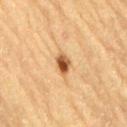Recorded during total-body skin imaging; not selected for excision or biopsy. A male patient in their mid-80s or older. The lesion is on the back. A 15 mm close-up tile from a total-body photography series done for melanoma screening.